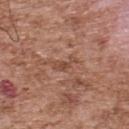location: the upper back
lesion diameter: ≈3 mm
patient: male, aged 53 to 57
image source: ~15 mm crop, total-body skin-cancer survey
tile lighting: white-light